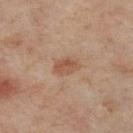Approximately 3 mm at its widest. A close-up tile cropped from a whole-body skin photograph, about 15 mm across. The lesion is located on the right lower leg. This is a cross-polarized tile. The patient is a female aged 53–57.